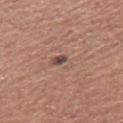follow-up = total-body-photography surveillance lesion; no biopsy
illumination = white-light
location = the right thigh
patient = female, aged 48–52
diameter = ≈2.5 mm
image = ~15 mm tile from a whole-body skin photo